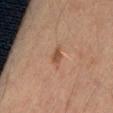Q: Was a biopsy performed?
A: imaged on a skin check; not biopsied
Q: How was this image acquired?
A: ~15 mm tile from a whole-body skin photo
Q: Patient demographics?
A: male, aged 53 to 57
Q: Lesion size?
A: ≈2 mm
Q: What is the anatomic site?
A: the left thigh